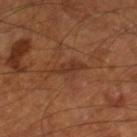Captured during whole-body skin photography for melanoma surveillance; the lesion was not biopsied. The recorded lesion diameter is about 4 mm. A 15 mm close-up tile from a total-body photography series done for melanoma screening. This is a cross-polarized tile. From the left leg. A male subject aged approximately 65.